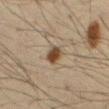Clinical impression:
Captured during whole-body skin photography for melanoma surveillance; the lesion was not biopsied.
Background:
A male patient, about 60 years old. A lesion tile, about 15 mm wide, cut from a 3D total-body photograph. From the abdomen.